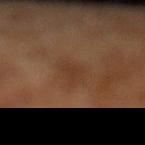notes: catalogued during a skin exam; not biopsied | image: ~15 mm crop, total-body skin-cancer survey | tile lighting: cross-polarized illumination | automated metrics: a border-irregularity index near 4/10, a color-variation rating of about 1/10, and radial color variation of about 0.5 | patient: male, about 70 years old | lesion diameter: about 4 mm | anatomic site: the left lower leg.